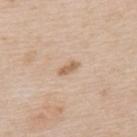notes: total-body-photography surveillance lesion; no biopsy
lesion diameter: about 3 mm
TBP lesion metrics: a lesion area of about 3 mm², an eccentricity of roughly 0.9, and a symmetry-axis asymmetry near 0.3; a lesion color around L≈63 a*≈17 b*≈32 in CIELAB, roughly 10 lightness units darker than nearby skin, and a normalized border contrast of about 7; a nevus-likeness score of about 15/100
subject: male, in their mid- to late 60s
location: the upper back
acquisition: total-body-photography crop, ~15 mm field of view